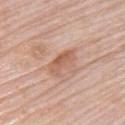notes = no biopsy performed (imaged during a skin exam)
automated metrics = a border-irregularity rating of about 3.5/10, internal color variation of about 3.5 on a 0–10 scale, and a peripheral color-asymmetry measure near 1; a classifier nevus-likeness of about 0/100 and lesion-presence confidence of about 100/100
lesion size = ~4 mm (longest diameter)
subject = female, in their mid- to late 60s
image source = ~15 mm crop, total-body skin-cancer survey
tile lighting = white-light
site = the upper back